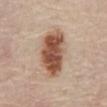Clinical impression: Imaged during a routine full-body skin examination; the lesion was not biopsied and no histopathology is available. Clinical summary: The lesion-visualizer software estimated an average lesion color of about L≈52 a*≈21 b*≈30 (CIELAB) and a normalized border contrast of about 11.5. The software also gave a border-irregularity rating of about 2/10 and a peripheral color-asymmetry measure near 2. The lesion is on the abdomen. A region of skin cropped from a whole-body photographic capture, roughly 15 mm wide. A male subject, aged 73–77. The recorded lesion diameter is about 6 mm.